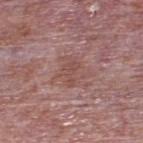biopsy status = catalogued during a skin exam; not biopsied
TBP lesion metrics = a lesion color around L≈48 a*≈22 b*≈23 in CIELAB, about 6 CIELAB-L* units darker than the surrounding skin, and a normalized border contrast of about 5.5
image = ~15 mm crop, total-body skin-cancer survey
subject = male, about 75 years old
lesion size = about 3 mm
lighting = white-light illumination
body site = the upper back